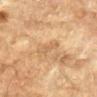Case summary:
• body site — the left forearm
• image — ~15 mm tile from a whole-body skin photo
• automated lesion analysis — a lesion–skin lightness drop of about 7; a border-irregularity rating of about 3.5/10, internal color variation of about 0.5 on a 0–10 scale, and radial color variation of about 0; a nevus-likeness score of about 0/100 and lesion-presence confidence of about 95/100
• size — about 3 mm
• patient — male, about 85 years old or older
• lighting — cross-polarized illumination